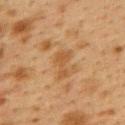This lesion was catalogued during total-body skin photography and was not selected for biopsy.
The lesion is located on the upper back.
This image is a 15 mm lesion crop taken from a total-body photograph.
Longest diameter approximately 3.5 mm.
The total-body-photography lesion software estimated an area of roughly 4.5 mm², an outline eccentricity of about 0.85 (0 = round, 1 = elongated), and two-axis asymmetry of about 0.65. It also reported a border-irregularity index near 6.5/10, a within-lesion color-variation index near 1/10, and radial color variation of about 0.5.
A female subject in their 40s.
This is a cross-polarized tile.